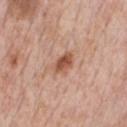The lesion was tiled from a total-body skin photograph and was not biopsied. The recorded lesion diameter is about 3 mm. A male subject, aged around 60. Cropped from a total-body skin-imaging series; the visible field is about 15 mm. On the chest.